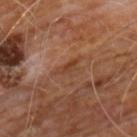Notes:
– site: the chest
– patient: male, approximately 60 years of age
– imaging modality: ~15 mm crop, total-body skin-cancer survey
– automated metrics: a footprint of about 3 mm², a shape eccentricity near 0.85, and a symmetry-axis asymmetry near 0.4; a color-variation rating of about 2/10 and peripheral color asymmetry of about 0.5
– lesion size: ~3 mm (longest diameter)
– lighting: cross-polarized illumination